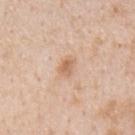Clinical impression: The lesion was photographed on a routine skin check and not biopsied; there is no pathology result. Image and clinical context: Captured under white-light illumination. A region of skin cropped from a whole-body photographic capture, roughly 15 mm wide. From the mid back. A male subject about 65 years old.